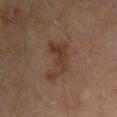Captured during whole-body skin photography for melanoma surveillance; the lesion was not biopsied. Cropped from a whole-body photographic skin survey; the tile spans about 15 mm. Captured under cross-polarized illumination. About 4.5 mm across. The lesion is located on the chest. The patient is a male in their mid-60s.